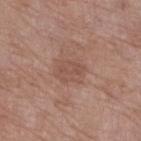Impression:
No biopsy was performed on this lesion — it was imaged during a full skin examination and was not determined to be concerning.
Image and clinical context:
Automated image analysis of the tile measured an outline eccentricity of about 0.65 (0 = round, 1 = elongated) and a symmetry-axis asymmetry near 0.2. And it measured a border-irregularity index near 2/10, a color-variation rating of about 2/10, and a peripheral color-asymmetry measure near 0.5. It also reported a lesion-detection confidence of about 100/100. Approximately 3.5 mm at its widest. Captured under white-light illumination. A female patient aged approximately 70. A roughly 15 mm field-of-view crop from a total-body skin photograph. From the leg.